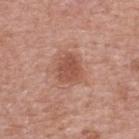Impression: The lesion was photographed on a routine skin check and not biopsied; there is no pathology result. Background: A 15 mm close-up extracted from a 3D total-body photography capture. Located on the upper back. A female subject, aged around 60.